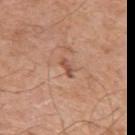Impression: Part of a total-body skin-imaging series; this lesion was reviewed on a skin check and was not flagged for biopsy. Context: This is a white-light tile. A male subject, in their mid-60s. The lesion's longest dimension is about 2.5 mm. A lesion tile, about 15 mm wide, cut from a 3D total-body photograph. The total-body-photography lesion software estimated a footprint of about 2.5 mm² and an eccentricity of roughly 0.9. The software also gave border irregularity of about 3 on a 0–10 scale, internal color variation of about 0 on a 0–10 scale, and a peripheral color-asymmetry measure near 0. From the left upper arm.